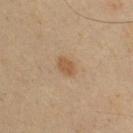Findings:
• workup · catalogued during a skin exam; not biopsied
• subject · male, roughly 35 years of age
• anatomic site · the upper back
• lighting · cross-polarized illumination
• lesion size · ~2.5 mm (longest diameter)
• image · ~15 mm tile from a whole-body skin photo